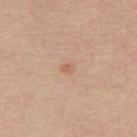{"biopsy_status": "not biopsied; imaged during a skin examination", "lesion_size": {"long_diameter_mm_approx": 1.5}, "site": "mid back", "lighting": "white-light", "patient": {"sex": "female", "age_approx": 55}, "image": {"source": "total-body photography crop", "field_of_view_mm": 15}}